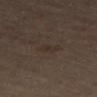{
  "biopsy_status": "not biopsied; imaged during a skin examination",
  "image": {
    "source": "total-body photography crop",
    "field_of_view_mm": 15
  },
  "patient": {
    "sex": "male",
    "age_approx": 65
  },
  "automated_metrics": {
    "area_mm2_approx": 4.0,
    "eccentricity": 0.8,
    "shape_asymmetry": 0.3
  },
  "lighting": "cross-polarized",
  "site": "leg"
}